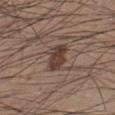Findings:
* notes: catalogued during a skin exam; not biopsied
* lesion size: ~3.5 mm (longest diameter)
* image: ~15 mm tile from a whole-body skin photo
* site: the left lower leg
* image-analysis metrics: border irregularity of about 2.5 on a 0–10 scale, a color-variation rating of about 3.5/10, and radial color variation of about 1
* patient: male, approximately 35 years of age